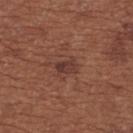Clinical impression: The lesion was tiled from a total-body skin photograph and was not biopsied. Acquisition and patient details: The lesion is located on the upper back. Measured at roughly 2.5 mm in maximum diameter. A 15 mm close-up extracted from a 3D total-body photography capture. The tile uses white-light illumination. A female patient, aged approximately 65. The lesion-visualizer software estimated a footprint of about 4 mm², a shape eccentricity near 0.75, and a symmetry-axis asymmetry near 0.25. It also reported a lesion–skin lightness drop of about 8 and a normalized lesion–skin contrast near 7.5. The software also gave border irregularity of about 3 on a 0–10 scale, internal color variation of about 4 on a 0–10 scale, and peripheral color asymmetry of about 1.5.